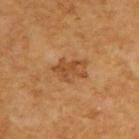follow-up: no biopsy performed (imaged during a skin exam)
automated metrics: a symmetry-axis asymmetry near 0.4; a detector confidence of about 100 out of 100 that the crop contains a lesion
image source: total-body-photography crop, ~15 mm field of view
patient: male, aged 58 to 62
lighting: cross-polarized
body site: the upper back